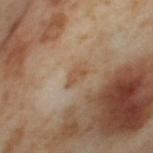Clinical impression:
Captured during whole-body skin photography for melanoma surveillance; the lesion was not biopsied.
Image and clinical context:
This image is a 15 mm lesion crop taken from a total-body photograph. The lesion is located on the left thigh. The patient is a female aged approximately 55.